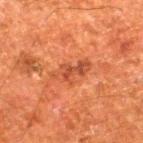biopsy status=total-body-photography surveillance lesion; no biopsy | acquisition=~15 mm tile from a whole-body skin photo | tile lighting=cross-polarized illumination | subject=male, aged 78 to 82 | body site=the right lower leg.